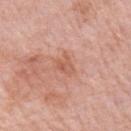| field | value |
|---|---|
| imaging modality | 15 mm crop, total-body photography |
| subject | female, aged approximately 70 |
| lesion diameter | ~2.5 mm (longest diameter) |
| location | the right upper arm |